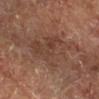Q: How large is the lesion?
A: ~7 mm (longest diameter)
Q: What is the anatomic site?
A: the right lower leg
Q: What is the imaging modality?
A: total-body-photography crop, ~15 mm field of view
Q: Patient demographics?
A: in their mid-60s